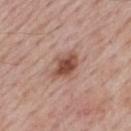This lesion was catalogued during total-body skin photography and was not selected for biopsy.